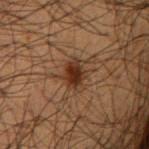Recorded during total-body skin imaging; not selected for excision or biopsy.
Cropped from a whole-body photographic skin survey; the tile spans about 15 mm.
A male patient aged 48 to 52.
The lesion's longest dimension is about 3.5 mm.
The lesion is located on the right upper arm.
Captured under cross-polarized illumination.